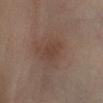workup: total-body-photography surveillance lesion; no biopsy | image: total-body-photography crop, ~15 mm field of view | diameter: ~2.5 mm (longest diameter) | location: the right leg | TBP lesion metrics: a lesion area of about 4.5 mm², an outline eccentricity of about 0.6 (0 = round, 1 = elongated), and a symmetry-axis asymmetry near 0.35; a border-irregularity index near 3.5/10, a color-variation rating of about 1.5/10, and radial color variation of about 0.5; a nevus-likeness score of about 0/100 and a detector confidence of about 100 out of 100 that the crop contains a lesion | subject: male, about 60 years old | illumination: cross-polarized.